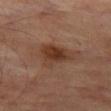biopsy status — total-body-photography surveillance lesion; no biopsy | patient — male, aged 68 to 72 | size — about 4.5 mm | acquisition — ~15 mm tile from a whole-body skin photo | anatomic site — the left thigh | TBP lesion metrics — roughly 9 lightness units darker than nearby skin; a classifier nevus-likeness of about 80/100 | tile lighting — cross-polarized.